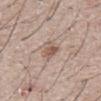| key | value |
|---|---|
| notes | total-body-photography surveillance lesion; no biopsy |
| lesion diameter | about 3 mm |
| subject | male, about 65 years old |
| location | the front of the torso |
| automated metrics | an outline eccentricity of about 0.8 (0 = round, 1 = elongated); an average lesion color of about L≈55 a*≈17 b*≈26 (CIELAB) and a normalized border contrast of about 7.5; border irregularity of about 2.5 on a 0–10 scale, internal color variation of about 2 on a 0–10 scale, and peripheral color asymmetry of about 0.5; a detector confidence of about 100 out of 100 that the crop contains a lesion |
| image | ~15 mm tile from a whole-body skin photo |